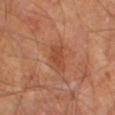Imaged during a routine full-body skin examination; the lesion was not biopsied and no histopathology is available. The patient is a male aged 63–67. From the left thigh. The lesion's longest dimension is about 3 mm. A region of skin cropped from a whole-body photographic capture, roughly 15 mm wide.